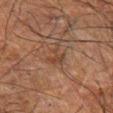A close-up tile cropped from a whole-body skin photograph, about 15 mm across.
A male patient aged 68 to 72.
On the left forearm.